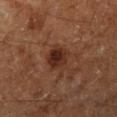Q: Patient demographics?
A: male, about 60 years old
Q: What is the lesion's diameter?
A: about 3.5 mm
Q: What kind of image is this?
A: 15 mm crop, total-body photography
Q: What is the anatomic site?
A: the right lower leg
Q: Automated lesion metrics?
A: a lesion color around L≈26 a*≈19 b*≈24 in CIELAB, a lesion–skin lightness drop of about 9, and a lesion-to-skin contrast of about 9.5 (normalized; higher = more distinct)